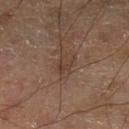biopsy_status: not biopsied; imaged during a skin examination
site: left lower leg
patient:
  sex: male
  age_approx: 70
image:
  source: total-body photography crop
  field_of_view_mm: 15